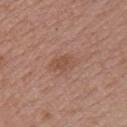The lesion was tiled from a total-body skin photograph and was not biopsied. A 15 mm crop from a total-body photograph taken for skin-cancer surveillance. On the right upper arm. The subject is a female aged 48 to 52.